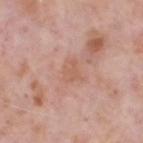{
  "patient": {
    "sex": "male",
    "age_approx": 70
  },
  "automated_metrics": {
    "cielab_L": 59,
    "cielab_a": 22,
    "cielab_b": 30,
    "vs_skin_darker_L": 6.0,
    "vs_skin_contrast_norm": 5.5,
    "border_irregularity_0_10": 3.5,
    "color_variation_0_10": 1.0,
    "peripheral_color_asymmetry": 0.5
  },
  "lesion_size": {
    "long_diameter_mm_approx": 2.5
  },
  "lighting": "white-light",
  "image": {
    "source": "total-body photography crop",
    "field_of_view_mm": 15
  },
  "site": "head or neck"
}